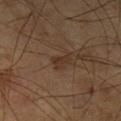Imaged during a routine full-body skin examination; the lesion was not biopsied and no histopathology is available. A 15 mm close-up tile from a total-body photography series done for melanoma screening. From the left lower leg. The patient is a male in their mid-60s. Imaged with cross-polarized lighting. An algorithmic analysis of the crop reported an average lesion color of about L≈30 a*≈15 b*≈25 (CIELAB), a lesion–skin lightness drop of about 6, and a lesion-to-skin contrast of about 6.5 (normalized; higher = more distinct). About 2.5 mm across.